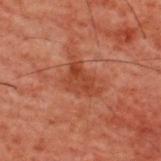{
  "biopsy_status": "not biopsied; imaged during a skin examination",
  "automated_metrics": {
    "area_mm2_approx": 9.0,
    "eccentricity": 0.75,
    "shape_asymmetry": 0.45
  },
  "lesion_size": {
    "long_diameter_mm_approx": 4.5
  },
  "image": {
    "source": "total-body photography crop",
    "field_of_view_mm": 15
  },
  "site": "upper back",
  "lighting": "cross-polarized",
  "patient": {
    "sex": "male",
    "age_approx": 60
  }
}